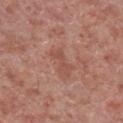<record>
  <biopsy_status>not biopsied; imaged during a skin examination</biopsy_status>
  <image>
    <source>total-body photography crop</source>
    <field_of_view_mm>15</field_of_view_mm>
  </image>
  <site>right lower leg</site>
  <lesion_size>
    <long_diameter_mm_approx>3.5</long_diameter_mm_approx>
  </lesion_size>
  <lighting>white-light</lighting>
  <patient>
    <sex>male</sex>
    <age_approx>55</age_approx>
  </patient>
</record>